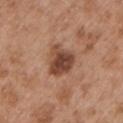workup = imaged on a skin check; not biopsied | lesion diameter = about 4 mm | subject = male, aged around 55 | site = the arm | image-analysis metrics = a border-irregularity rating of about 3/10 and peripheral color asymmetry of about 1.5; a nevus-likeness score of about 10/100 and a lesion-detection confidence of about 100/100 | tile lighting = white-light | image source = ~15 mm tile from a whole-body skin photo.